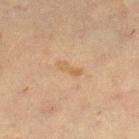Clinical impression: Captured during whole-body skin photography for melanoma surveillance; the lesion was not biopsied. Context: A region of skin cropped from a whole-body photographic capture, roughly 15 mm wide. A female patient, aged approximately 40. The lesion is located on the right lower leg.